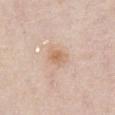Assessment:
This lesion was catalogued during total-body skin photography and was not selected for biopsy.
Acquisition and patient details:
Cropped from a total-body skin-imaging series; the visible field is about 15 mm. The lesion's longest dimension is about 3 mm. The patient is a female aged approximately 60. Captured under white-light illumination. The lesion is located on the abdomen.